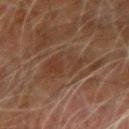Impression:
The lesion was tiled from a total-body skin photograph and was not biopsied.
Clinical summary:
This image is a 15 mm lesion crop taken from a total-body photograph. This is a cross-polarized tile. Automated tile analysis of the lesion measured a footprint of about 15 mm² and a shape eccentricity near 0.8. The analysis additionally found a lesion color around L≈29 a*≈14 b*≈22 in CIELAB, about 4 CIELAB-L* units darker than the surrounding skin, and a normalized border contrast of about 4.5. The analysis additionally found a border-irregularity rating of about 4/10, internal color variation of about 4 on a 0–10 scale, and radial color variation of about 1. The lesion is located on the left forearm. Approximately 6 mm at its widest. A male patient, approximately 80 years of age.